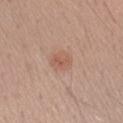The lesion was tiled from a total-body skin photograph and was not biopsied. Captured under white-light illumination. The patient is a male aged 48–52. The lesion's longest dimension is about 3 mm. Automated tile analysis of the lesion measured a lesion area of about 6 mm², a shape eccentricity near 0.55, and two-axis asymmetry of about 0.15. The analysis additionally found a lesion color around L≈57 a*≈21 b*≈28 in CIELAB, about 7 CIELAB-L* units darker than the surrounding skin, and a normalized lesion–skin contrast near 5.5. The analysis additionally found a border-irregularity index near 1.5/10, a color-variation rating of about 3/10, and radial color variation of about 1. The analysis additionally found a nevus-likeness score of about 65/100 and a detector confidence of about 100 out of 100 that the crop contains a lesion. A close-up tile cropped from a whole-body skin photograph, about 15 mm across. On the left forearm.